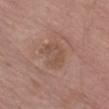Assessment:
The lesion was photographed on a routine skin check and not biopsied; there is no pathology result.
Context:
The tile uses white-light illumination. About 3.5 mm across. Located on the left thigh. A lesion tile, about 15 mm wide, cut from a 3D total-body photograph. The subject is a male roughly 65 years of age. The lesion-visualizer software estimated a lesion area of about 6.5 mm², a shape eccentricity near 0.65, and a shape-asymmetry score of about 0.45 (0 = symmetric).